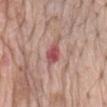No biopsy was performed on this lesion — it was imaged during a full skin examination and was not determined to be concerning. The subject is a female aged around 75. The lesion is on the mid back. The tile uses white-light illumination. A close-up tile cropped from a whole-body skin photograph, about 15 mm across. Approximately 3 mm at its widest.